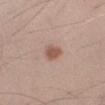Clinical impression:
Recorded during total-body skin imaging; not selected for excision or biopsy.
Clinical summary:
A male subject, aged 43 to 47. A 15 mm crop from a total-body photograph taken for skin-cancer surveillance. Measured at roughly 2.5 mm in maximum diameter. This is a white-light tile. On the right forearm.